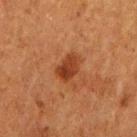No biopsy was performed on this lesion — it was imaged during a full skin examination and was not determined to be concerning. The recorded lesion diameter is about 3.5 mm. A 15 mm close-up extracted from a 3D total-body photography capture. The lesion-visualizer software estimated a border-irregularity index near 2/10 and radial color variation of about 1.5. On the right upper arm. Captured under cross-polarized illumination. A female subject, roughly 50 years of age.